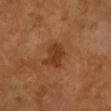Impression:
Captured during whole-body skin photography for melanoma surveillance; the lesion was not biopsied.
Acquisition and patient details:
Longest diameter approximately 3.5 mm. On the left arm. An algorithmic analysis of the crop reported a symmetry-axis asymmetry near 0.3. The analysis additionally found a lesion color around L≈39 a*≈25 b*≈36 in CIELAB and a normalized border contrast of about 7.5. The software also gave a nevus-likeness score of about 50/100. The tile uses cross-polarized illumination. A female patient about 60 years old. A 15 mm close-up tile from a total-body photography series done for melanoma screening.